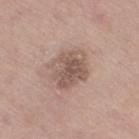This lesion was catalogued during total-body skin photography and was not selected for biopsy. A male patient approximately 75 years of age. The lesion is located on the right thigh. A 15 mm close-up extracted from a 3D total-body photography capture. Measured at roughly 4.5 mm in maximum diameter.